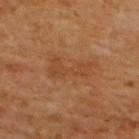Findings:
- biopsy status: no biopsy performed (imaged during a skin exam)
- patient: male, approximately 65 years of age
- diameter: ≈5.5 mm
- body site: the back
- tile lighting: cross-polarized illumination
- image source: ~15 mm tile from a whole-body skin photo